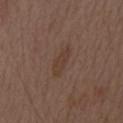Q: Is there a histopathology result?
A: catalogued during a skin exam; not biopsied
Q: Lesion size?
A: ~4 mm (longest diameter)
Q: What are the patient's age and sex?
A: male, aged around 70
Q: How was the tile lit?
A: white-light illumination
Q: What kind of image is this?
A: total-body-photography crop, ~15 mm field of view
Q: What is the anatomic site?
A: the mid back